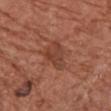Q: Was a biopsy performed?
A: no biopsy performed (imaged during a skin exam)
Q: What is the anatomic site?
A: the chest
Q: How was the tile lit?
A: white-light illumination
Q: What kind of image is this?
A: 15 mm crop, total-body photography
Q: Who is the patient?
A: female, approximately 75 years of age